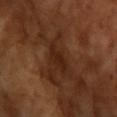| key | value |
|---|---|
| biopsy status | catalogued during a skin exam; not biopsied |
| size | about 3 mm |
| imaging modality | total-body-photography crop, ~15 mm field of view |
| illumination | cross-polarized |
| automated metrics | a lesion area of about 3.5 mm² and an outline eccentricity of about 0.85 (0 = round, 1 = elongated); a lesion color around L≈22 a*≈20 b*≈26 in CIELAB, roughly 6 lightness units darker than nearby skin, and a normalized lesion–skin contrast near 7 |
| subject | male, aged 63 to 67 |